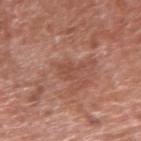  biopsy_status: not biopsied; imaged during a skin examination
  patient:
    sex: male
    age_approx: 80
  image:
    source: total-body photography crop
    field_of_view_mm: 15
  site: arm